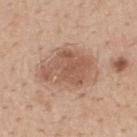The lesion was photographed on a routine skin check and not biopsied; there is no pathology result. This is a white-light tile. The recorded lesion diameter is about 6 mm. A 15 mm crop from a total-body photograph taken for skin-cancer surveillance. The lesion is on the upper back. A male patient aged 58–62. The lesion-visualizer software estimated internal color variation of about 3 on a 0–10 scale and a peripheral color-asymmetry measure near 1.